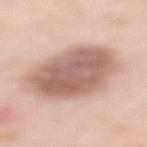Clinical impression:
Imaged during a routine full-body skin examination; the lesion was not biopsied and no histopathology is available.
Clinical summary:
The tile uses white-light illumination. The total-body-photography lesion software estimated an average lesion color of about L≈62 a*≈20 b*≈25 (CIELAB) and a lesion–skin lightness drop of about 15. From the upper back. A female subject, aged approximately 50. A 15 mm close-up extracted from a 3D total-body photography capture. The recorded lesion diameter is about 8.5 mm.